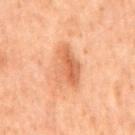Q: Is there a histopathology result?
A: no biopsy performed (imaged during a skin exam)
Q: What is the lesion's diameter?
A: about 5 mm
Q: Lesion location?
A: the back
Q: Patient demographics?
A: male, roughly 70 years of age
Q: How was the tile lit?
A: cross-polarized illumination
Q: What is the imaging modality?
A: 15 mm crop, total-body photography